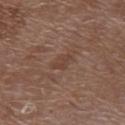notes — catalogued during a skin exam; not biopsied | image-analysis metrics — a lesion area of about 3 mm², an outline eccentricity of about 0.85 (0 = round, 1 = elongated), and a symmetry-axis asymmetry near 0.45; a nevus-likeness score of about 0/100 | lesion diameter — ~2.5 mm (longest diameter) | site — the upper back | subject — female, aged 68 to 72 | image source — ~15 mm crop, total-body skin-cancer survey.